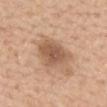notes: imaged on a skin check; not biopsied
patient: female, aged approximately 65
body site: the back
image source: total-body-photography crop, ~15 mm field of view
diameter: ~5 mm (longest diameter)
illumination: white-light